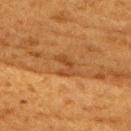No biopsy was performed on this lesion — it was imaged during a full skin examination and was not determined to be concerning. About 2.5 mm across. The tile uses cross-polarized illumination. A female subject aged approximately 50. This image is a 15 mm lesion crop taken from a total-body photograph. On the back.